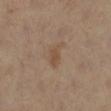follow-up=catalogued during a skin exam; not biopsied | body site=the right lower leg | patient=female, in their 40s | acquisition=total-body-photography crop, ~15 mm field of view | illumination=cross-polarized.